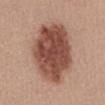Measured at roughly 8.5 mm in maximum diameter.
The lesion is located on the abdomen.
A female subject aged 43–47.
Automated image analysis of the tile measured a shape eccentricity near 0.7 and two-axis asymmetry of about 0.15. The analysis additionally found a mean CIELAB color near L≈48 a*≈23 b*≈27, a lesion–skin lightness drop of about 16, and a normalized lesion–skin contrast near 11.5.
A close-up tile cropped from a whole-body skin photograph, about 15 mm across.
The tile uses white-light illumination.